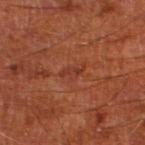Findings:
• workup — no biopsy performed (imaged during a skin exam)
• size — ≈3 mm
• body site — the left lower leg
• subject — male, aged 63–67
• acquisition — total-body-photography crop, ~15 mm field of view
• automated metrics — an area of roughly 3 mm² and an eccentricity of roughly 0.9; a lesion color around L≈35 a*≈27 b*≈31 in CIELAB, about 6 CIELAB-L* units darker than the surrounding skin, and a normalized border contrast of about 5; a classifier nevus-likeness of about 0/100